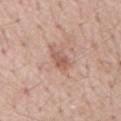<tbp_lesion>
<biopsy_status>not biopsied; imaged during a skin examination</biopsy_status>
<site>front of the torso</site>
<image>
  <source>total-body photography crop</source>
  <field_of_view_mm>15</field_of_view_mm>
</image>
<patient>
  <sex>male</sex>
  <age_approx>60</age_approx>
</patient>
</tbp_lesion>